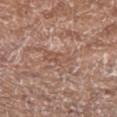Imaged with white-light lighting. A region of skin cropped from a whole-body photographic capture, roughly 15 mm wide. On the right forearm. A female subject roughly 75 years of age.